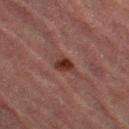biopsy status: no biopsy performed (imaged during a skin exam) | anatomic site: the right thigh | illumination: cross-polarized | patient: female, roughly 70 years of age | image source: 15 mm crop, total-body photography | image-analysis metrics: border irregularity of about 2 on a 0–10 scale, a color-variation rating of about 3/10, and peripheral color asymmetry of about 1; a classifier nevus-likeness of about 95/100 and lesion-presence confidence of about 100/100.